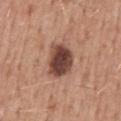The lesion was tiled from a total-body skin photograph and was not biopsied. A lesion tile, about 15 mm wide, cut from a 3D total-body photograph. Captured under white-light illumination. Automated image analysis of the tile measured an eccentricity of roughly 0.55 and a shape-asymmetry score of about 0.2 (0 = symmetric). It also reported roughly 18 lightness units darker than nearby skin and a lesion-to-skin contrast of about 13 (normalized; higher = more distinct). The software also gave border irregularity of about 2 on a 0–10 scale. From the mid back. A male subject, approximately 55 years of age. The lesion's longest dimension is about 4 mm.